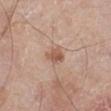Assessment:
Part of a total-body skin-imaging series; this lesion was reviewed on a skin check and was not flagged for biopsy.
Clinical summary:
A male subject, aged 78–82. An algorithmic analysis of the crop reported a footprint of about 4 mm² and a shape-asymmetry score of about 0.2 (0 = symmetric). And it measured a lesion color around L≈56 a*≈20 b*≈29 in CIELAB and a lesion–skin lightness drop of about 10. The software also gave internal color variation of about 3 on a 0–10 scale and peripheral color asymmetry of about 1.5. The software also gave a classifier nevus-likeness of about 55/100 and a detector confidence of about 100 out of 100 that the crop contains a lesion. This is a white-light tile. About 2.5 mm across. Located on the right leg. A 15 mm crop from a total-body photograph taken for skin-cancer surveillance.